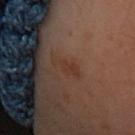The lesion was photographed on a routine skin check and not biopsied; there is no pathology result.
Cropped from a total-body skin-imaging series; the visible field is about 15 mm.
The patient is a female aged 58–62.
Longest diameter approximately 3.5 mm.
From the front of the torso.
Captured under cross-polarized illumination.